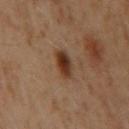Imaged during a routine full-body skin examination; the lesion was not biopsied and no histopathology is available. Cropped from a whole-body photographic skin survey; the tile spans about 15 mm. An algorithmic analysis of the crop reported a border-irregularity rating of about 1.5/10, a within-lesion color-variation index near 5/10, and radial color variation of about 1.5. The tile uses cross-polarized illumination. The subject is a male aged approximately 60. On the mid back. Longest diameter approximately 3.5 mm.